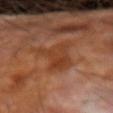| field | value |
|---|---|
| workup | no biopsy performed (imaged during a skin exam) |